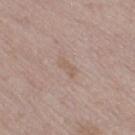Clinical impression: This lesion was catalogued during total-body skin photography and was not selected for biopsy. Context: The total-body-photography lesion software estimated a footprint of about 3 mm², an eccentricity of roughly 0.9, and two-axis asymmetry of about 0.35. The software also gave border irregularity of about 3.5 on a 0–10 scale, a color-variation rating of about 0/10, and a peripheral color-asymmetry measure near 0. A female subject, aged 38 to 42. This image is a 15 mm lesion crop taken from a total-body photograph. Approximately 2.5 mm at its widest. The lesion is on the right thigh. Captured under white-light illumination.